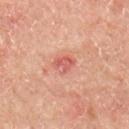  biopsy_status: not biopsied; imaged during a skin examination
  patient:
    sex: male
    age_approx: 65
  image:
    source: total-body photography crop
    field_of_view_mm: 15
  automated_metrics:
    border_irregularity_0_10: 2.5
    color_variation_0_10: 4.0
    nevus_likeness_0_100: 0
    lesion_detection_confidence_0_100: 100
  lighting: cross-polarized
  site: right upper arm
  lesion_size:
    long_diameter_mm_approx: 3.0